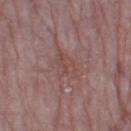workup: imaged on a skin check; not biopsied
TBP lesion metrics: an area of roughly 6 mm², an eccentricity of roughly 0.65, and a shape-asymmetry score of about 0.4 (0 = symmetric); a lesion color around L≈47 a*≈20 b*≈21 in CIELAB and roughly 5 lightness units darker than nearby skin
subject: female, about 65 years old
anatomic site: the leg
acquisition: ~15 mm crop, total-body skin-cancer survey